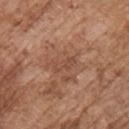Assessment:
Captured during whole-body skin photography for melanoma surveillance; the lesion was not biopsied.
Image and clinical context:
Imaged with white-light lighting. A male subject aged around 75. The lesion is on the chest. The lesion-visualizer software estimated an area of roughly 3 mm² and two-axis asymmetry of about 0.3. It also reported a lesion–skin lightness drop of about 6. A roughly 15 mm field-of-view crop from a total-body skin photograph.